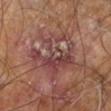Notes:
• follow-up: imaged on a skin check; not biopsied
• acquisition: ~15 mm tile from a whole-body skin photo
• patient: male, aged 58 to 62
• anatomic site: the right leg